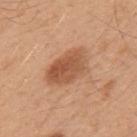The lesion was tiled from a total-body skin photograph and was not biopsied. Located on the mid back. Imaged with white-light lighting. A region of skin cropped from a whole-body photographic capture, roughly 15 mm wide. About 5.5 mm across. A male patient, aged around 50.